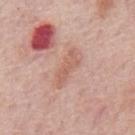Imaged during a routine full-body skin examination; the lesion was not biopsied and no histopathology is available.
About 4.5 mm across.
Automated image analysis of the tile measured an area of roughly 6.5 mm², an eccentricity of roughly 0.95, and two-axis asymmetry of about 0.3. The software also gave a border-irregularity index near 4/10 and peripheral color asymmetry of about 0.5.
A close-up tile cropped from a whole-body skin photograph, about 15 mm across.
The patient is a male in their mid- to late 70s.
This is a white-light tile.
Located on the back.